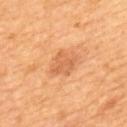biopsy status = no biopsy performed (imaged during a skin exam) | site = the upper back | TBP lesion metrics = a border-irregularity rating of about 2.5/10 and a within-lesion color-variation index near 2/10; a classifier nevus-likeness of about 65/100 and a detector confidence of about 100 out of 100 that the crop contains a lesion | patient = male, approximately 65 years of age | lesion diameter = ~3.5 mm (longest diameter) | image source = ~15 mm crop, total-body skin-cancer survey | lighting = cross-polarized illumination.